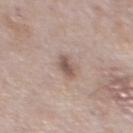{"biopsy_status": "not biopsied; imaged during a skin examination", "patient": {"sex": "male", "age_approx": 75}, "lesion_size": {"long_diameter_mm_approx": 3.0}, "image": {"source": "total-body photography crop", "field_of_view_mm": 15}, "lighting": "white-light", "site": "chest"}